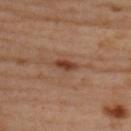Recorded during total-body skin imaging; not selected for excision or biopsy.
A roughly 15 mm field-of-view crop from a total-body skin photograph.
A female subject, about 40 years old.
Imaged with cross-polarized lighting.
From the back.